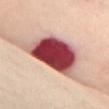Recorded during total-body skin imaging; not selected for excision or biopsy.
This is a cross-polarized tile.
A 15 mm close-up tile from a total-body photography series done for melanoma screening.
The lesion is located on the front of the torso.
Longest diameter approximately 8.5 mm.
A female patient, in their mid- to late 50s.